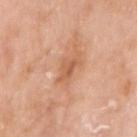<record>
<biopsy_status>not biopsied; imaged during a skin examination</biopsy_status>
<lesion_size>
  <long_diameter_mm_approx>4.0</long_diameter_mm_approx>
</lesion_size>
<patient>
  <sex>male</sex>
  <age_approx>80</age_approx>
</patient>
<site>right upper arm</site>
<lighting>white-light</lighting>
<image>
  <source>total-body photography crop</source>
  <field_of_view_mm>15</field_of_view_mm>
</image>
<automated_metrics>
  <area_mm2_approx>6.5</area_mm2_approx>
  <eccentricity>0.8</eccentricity>
  <shape_asymmetry>0.35</shape_asymmetry>
  <cielab_L>60</cielab_L>
  <cielab_a>24</cielab_a>
  <cielab_b>36</cielab_b>
  <vs_skin_contrast_norm>5.5</vs_skin_contrast_norm>
  <nevus_likeness_0_100>0</nevus_likeness_0_100>
  <lesion_detection_confidence_0_100>100</lesion_detection_confidence_0_100>
</automated_metrics>
</record>